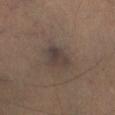{"automated_metrics": {"area_mm2_approx": 7.0, "eccentricity": 0.7, "cielab_L": 36, "cielab_a": 11, "cielab_b": 17, "vs_skin_darker_L": 7.0, "vs_skin_contrast_norm": 7.5, "color_variation_0_10": 3.5, "peripheral_color_asymmetry": 1.0, "nevus_likeness_0_100": 0, "lesion_detection_confidence_0_100": 90}, "lighting": "cross-polarized", "image": {"source": "total-body photography crop", "field_of_view_mm": 15}, "patient": {"sex": "male", "age_approx": 35}, "site": "left leg", "lesion_size": {"long_diameter_mm_approx": 3.5}}